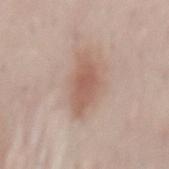No biopsy was performed on this lesion — it was imaged during a full skin examination and was not determined to be concerning. The lesion's longest dimension is about 6 mm. This is a white-light tile. A female subject roughly 50 years of age. From the back. A 15 mm crop from a total-body photograph taken for skin-cancer surveillance.